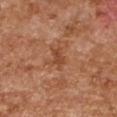| key | value |
|---|---|
| follow-up | imaged on a skin check; not biopsied |
| lighting | cross-polarized |
| site | the arm |
| subject | female, aged 48–52 |
| image | ~15 mm crop, total-body skin-cancer survey |